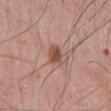workup: total-body-photography surveillance lesion; no biopsy | TBP lesion metrics: an area of roughly 4.5 mm², an outline eccentricity of about 0.75 (0 = round, 1 = elongated), and a symmetry-axis asymmetry near 0.25 | image source: 15 mm crop, total-body photography | illumination: white-light | lesion size: ~2.5 mm (longest diameter) | anatomic site: the mid back | patient: male, about 70 years old.